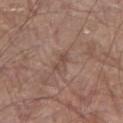{"biopsy_status": "not biopsied; imaged during a skin examination", "patient": {"sex": "male", "age_approx": 80}, "automated_metrics": {"area_mm2_approx": 2.5, "eccentricity": 0.9, "shape_asymmetry": 0.3, "border_irregularity_0_10": 3.5, "color_variation_0_10": 0.0}, "site": "left lower leg", "image": {"source": "total-body photography crop", "field_of_view_mm": 15}, "lighting": "white-light"}